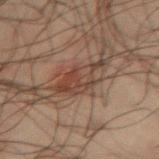<case>
<patient>
  <sex>male</sex>
  <age_approx>50</age_approx>
</patient>
<site>right thigh</site>
<image>
  <source>total-body photography crop</source>
  <field_of_view_mm>15</field_of_view_mm>
</image>
<lesion_size>
  <long_diameter_mm_approx>5.5</long_diameter_mm_approx>
</lesion_size>
<lighting>cross-polarized</lighting>
<automated_metrics>
  <border_irregularity_0_10>5.0</border_irregularity_0_10>
  <color_variation_0_10>4.5</color_variation_0_10>
  <peripheral_color_asymmetry>1.5</peripheral_color_asymmetry>
</automated_metrics>
</case>